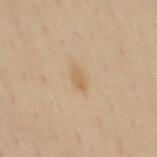  biopsy_status: not biopsied; imaged during a skin examination
  automated_metrics:
    area_mm2_approx: 3.5
    eccentricity: 0.8
    shape_asymmetry: 0.35
    cielab_L: 61
    cielab_a: 13
    cielab_b: 36
    vs_skin_darker_L: 6.0
    border_irregularity_0_10: 3.0
    color_variation_0_10: 2.5
    peripheral_color_asymmetry: 1.0
    nevus_likeness_0_100: 5
    lesion_detection_confidence_0_100: 100
  site: mid back
  lesion_size:
    long_diameter_mm_approx: 2.5
  image:
    source: total-body photography crop
    field_of_view_mm: 15
  patient:
    sex: male
    age_approx: 45